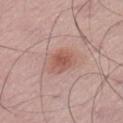No biopsy was performed on this lesion — it was imaged during a full skin examination and was not determined to be concerning. From the left upper arm. This image is a 15 mm lesion crop taken from a total-body photograph. A male patient, aged 48 to 52.